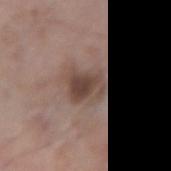The lesion was tiled from a total-body skin photograph and was not biopsied. From the left forearm. Captured under white-light illumination. Measured at roughly 4 mm in maximum diameter. A lesion tile, about 15 mm wide, cut from a 3D total-body photograph. The total-body-photography lesion software estimated a lesion color around L≈45 a*≈16 b*≈22 in CIELAB, a lesion–skin lightness drop of about 10, and a normalized lesion–skin contrast near 8. The analysis additionally found a border-irregularity index near 3/10, a color-variation rating of about 5/10, and a peripheral color-asymmetry measure near 1.5. And it measured a nevus-likeness score of about 30/100. A male subject approximately 60 years of age.